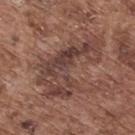follow-up = no biopsy performed (imaged during a skin exam)
patient = male, aged around 75
illumination = white-light
imaging modality = 15 mm crop, total-body photography
location = the back